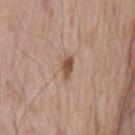Imaged during a routine full-body skin examination; the lesion was not biopsied and no histopathology is available. The total-body-photography lesion software estimated a lesion color around L≈51 a*≈20 b*≈28 in CIELAB, roughly 13 lightness units darker than nearby skin, and a lesion-to-skin contrast of about 9 (normalized; higher = more distinct). It also reported a border-irregularity rating of about 3.5/10, internal color variation of about 1.5 on a 0–10 scale, and a peripheral color-asymmetry measure near 0.5. A male patient aged approximately 65. On the chest. A 15 mm close-up extracted from a 3D total-body photography capture.